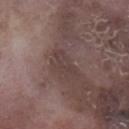notes = no biopsy performed (imaged during a skin exam)
subject = male, about 75 years old
image-analysis metrics = an average lesion color of about L≈39 a*≈15 b*≈18 (CIELAB), about 5 CIELAB-L* units darker than the surrounding skin, and a normalized border contrast of about 4.5; a nevus-likeness score of about 0/100 and a lesion-detection confidence of about 75/100
anatomic site = the left lower leg
image source = ~15 mm tile from a whole-body skin photo
lighting = white-light illumination